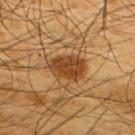| feature | finding |
|---|---|
| biopsy status | no biopsy performed (imaged during a skin exam) |
| patient | male, in their mid-60s |
| automated metrics | a footprint of about 11 mm², an eccentricity of roughly 0.75, and a shape-asymmetry score of about 0.15 (0 = symmetric); a within-lesion color-variation index near 4.5/10 and radial color variation of about 1.5; a nevus-likeness score of about 95/100 and a lesion-detection confidence of about 100/100 |
| lighting | cross-polarized |
| size | ≈4.5 mm |
| imaging modality | ~15 mm crop, total-body skin-cancer survey |
| site | the right upper arm |